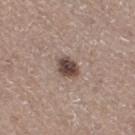Assessment: Captured during whole-body skin photography for melanoma surveillance; the lesion was not biopsied. Image and clinical context: Cropped from a total-body skin-imaging series; the visible field is about 15 mm. On the right thigh. Measured at roughly 3 mm in maximum diameter. Imaged with white-light lighting. The patient is a female in their 50s.